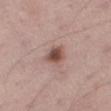Context: Longest diameter approximately 3 mm. A male subject, in their mid- to late 70s. A 15 mm crop from a total-body photograph taken for skin-cancer surveillance. Located on the right thigh. The tile uses white-light illumination.